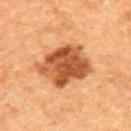workup: total-body-photography surveillance lesion; no biopsy | size: about 6.5 mm | image-analysis metrics: an outline eccentricity of about 0.7 (0 = round, 1 = elongated) and two-axis asymmetry of about 0.25; a border-irregularity index near 3/10 | location: the upper back | subject: male, in their 50s | lighting: cross-polarized illumination | image: ~15 mm tile from a whole-body skin photo.